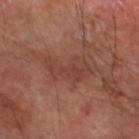<case>
  <biopsy_status>not biopsied; imaged during a skin examination</biopsy_status>
  <patient>
    <sex>male</sex>
    <age_approx>70</age_approx>
  </patient>
  <image>
    <source>total-body photography crop</source>
    <field_of_view_mm>15</field_of_view_mm>
  </image>
  <lesion_size>
    <long_diameter_mm_approx>5.5</long_diameter_mm_approx>
  </lesion_size>
  <automated_metrics>
    <cielab_L>40</cielab_L>
    <cielab_a>23</cielab_a>
    <cielab_b>26</cielab_b>
    <vs_skin_darker_L>6.0</vs_skin_darker_L>
    <vs_skin_contrast_norm>5.0</vs_skin_contrast_norm>
    <color_variation_0_10>3.0</color_variation_0_10>
    <peripheral_color_asymmetry>1.0</peripheral_color_asymmetry>
    <nevus_likeness_0_100>0</nevus_likeness_0_100>
    <lesion_detection_confidence_0_100>100</lesion_detection_confidence_0_100>
  </automated_metrics>
  <lighting>cross-polarized</lighting>
  <site>leg</site>
</case>